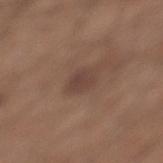{"image": {"source": "total-body photography crop", "field_of_view_mm": 15}, "site": "left lower leg", "lighting": "white-light", "patient": {"sex": "male", "age_approx": 30}}